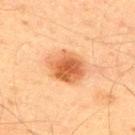No biopsy was performed on this lesion — it was imaged during a full skin examination and was not determined to be concerning. A close-up tile cropped from a whole-body skin photograph, about 15 mm across. The subject is a male in their mid-40s. Located on the right upper arm.